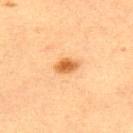Clinical summary:
A female patient approximately 65 years of age. From the upper back. Measured at roughly 3 mm in maximum diameter. This is a cross-polarized tile. This image is a 15 mm lesion crop taken from a total-body photograph.